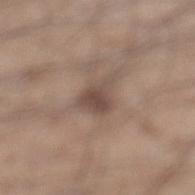Clinical impression:
No biopsy was performed on this lesion — it was imaged during a full skin examination and was not determined to be concerning.
Clinical summary:
The lesion is located on the left lower leg. A 15 mm crop from a total-body photograph taken for skin-cancer surveillance. Automated tile analysis of the lesion measured a lesion area of about 7 mm², an eccentricity of roughly 0.65, and a symmetry-axis asymmetry near 0.4. The software also gave a lesion color around L≈48 a*≈15 b*≈23 in CIELAB, a lesion–skin lightness drop of about 10, and a lesion-to-skin contrast of about 7.5 (normalized; higher = more distinct). It also reported a classifier nevus-likeness of about 30/100 and lesion-presence confidence of about 100/100. A male patient aged 33 to 37.